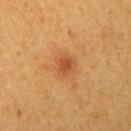{"biopsy_status": "not biopsied; imaged during a skin examination", "image": {"source": "total-body photography crop", "field_of_view_mm": 15}, "site": "right upper arm", "lesion_size": {"long_diameter_mm_approx": 2.5}, "patient": {"sex": "female", "age_approx": 40}, "lighting": "cross-polarized"}